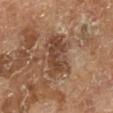workup: total-body-photography surveillance lesion; no biopsy
tile lighting: cross-polarized illumination
diameter: about 5 mm
site: the right lower leg
image-analysis metrics: an outline eccentricity of about 0.75 (0 = round, 1 = elongated); border irregularity of about 3.5 on a 0–10 scale, internal color variation of about 5 on a 0–10 scale, and a peripheral color-asymmetry measure near 1.5; a classifier nevus-likeness of about 0/100
subject: male, aged around 65
imaging modality: ~15 mm crop, total-body skin-cancer survey